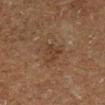Findings:
– workup · catalogued during a skin exam; not biopsied
– tile lighting · cross-polarized illumination
– patient · male, in their mid- to late 70s
– body site · the right lower leg
– diameter · about 3 mm
– image · 15 mm crop, total-body photography